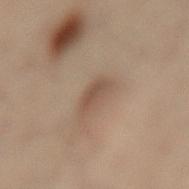Q: Was a biopsy performed?
A: no biopsy performed (imaged during a skin exam)
Q: What is the anatomic site?
A: the lower back
Q: What is the imaging modality?
A: 15 mm crop, total-body photography
Q: What is the lesion's diameter?
A: about 3 mm
Q: Who is the patient?
A: female, aged approximately 55